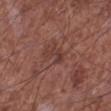No biopsy was performed on this lesion — it was imaged during a full skin examination and was not determined to be concerning.
A male patient about 65 years old.
The total-body-photography lesion software estimated an average lesion color of about L≈38 a*≈22 b*≈23 (CIELAB), roughly 7 lightness units darker than nearby skin, and a normalized lesion–skin contrast near 5.5. The software also gave a detector confidence of about 100 out of 100 that the crop contains a lesion.
The tile uses white-light illumination.
A close-up tile cropped from a whole-body skin photograph, about 15 mm across.
On the left lower leg.
Measured at roughly 3 mm in maximum diameter.